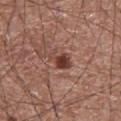* notes — catalogued during a skin exam; not biopsied
* diameter — about 3.5 mm
* site — the abdomen
* imaging modality — total-body-photography crop, ~15 mm field of view
* automated metrics — an area of roughly 5.5 mm², an outline eccentricity of about 0.75 (0 = round, 1 = elongated), and a shape-asymmetry score of about 0.3 (0 = symmetric)
* patient — male, approximately 75 years of age
* lighting — white-light illumination